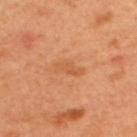Assessment: Recorded during total-body skin imaging; not selected for excision or biopsy. Context: About 3.5 mm across. A male patient, aged 48 to 52. Captured under cross-polarized illumination. The lesion is on the upper back. A 15 mm close-up extracted from a 3D total-body photography capture.